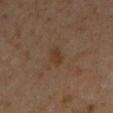From the chest. The tile uses cross-polarized illumination. A male subject aged 43–47. A roughly 15 mm field-of-view crop from a total-body skin photograph.